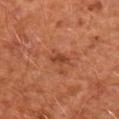{
  "biopsy_status": "not biopsied; imaged during a skin examination",
  "image": {
    "source": "total-body photography crop",
    "field_of_view_mm": 15
  },
  "lesion_size": {
    "long_diameter_mm_approx": 2.5
  },
  "patient": {
    "sex": "male",
    "age_approx": 65
  },
  "lighting": "cross-polarized",
  "site": "arm"
}